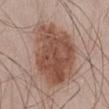Impression:
The lesion was photographed on a routine skin check and not biopsied; there is no pathology result.
Image and clinical context:
The total-body-photography lesion software estimated a lesion area of about 41 mm², an eccentricity of roughly 0.7, and two-axis asymmetry of about 0.15. And it measured a lesion color around L≈50 a*≈20 b*≈27 in CIELAB, a lesion–skin lightness drop of about 13, and a normalized lesion–skin contrast near 9.5. It also reported a classifier nevus-likeness of about 65/100 and a detector confidence of about 100 out of 100 that the crop contains a lesion. Captured under white-light illumination. A male patient, approximately 55 years of age. About 9 mm across. Cropped from a whole-body photographic skin survey; the tile spans about 15 mm. The lesion is located on the abdomen.